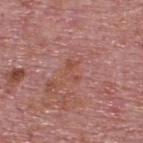Located on the back.
A close-up tile cropped from a whole-body skin photograph, about 15 mm across.
A male patient roughly 75 years of age.
Captured under white-light illumination.
Measured at roughly 2.5 mm in maximum diameter.